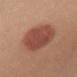workup: total-body-photography surveillance lesion; no biopsy | image-analysis metrics: a border-irregularity index near 1.5/10, a color-variation rating of about 3/10, and radial color variation of about 1 | lighting: white-light | patient: female, aged 33 to 37 | lesion diameter: ≈7 mm | site: the chest | imaging modality: ~15 mm crop, total-body skin-cancer survey.